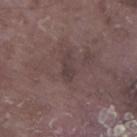Q: Was a biopsy performed?
A: imaged on a skin check; not biopsied
Q: How was this image acquired?
A: ~15 mm tile from a whole-body skin photo
Q: Lesion location?
A: the leg
Q: What is the lesion's diameter?
A: ≈3.5 mm
Q: What are the patient's age and sex?
A: male, approximately 75 years of age
Q: How was the tile lit?
A: white-light